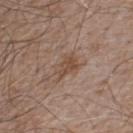Clinical impression:
This lesion was catalogued during total-body skin photography and was not selected for biopsy.
Image and clinical context:
Cropped from a total-body skin-imaging series; the visible field is about 15 mm. Captured under white-light illumination. The total-body-photography lesion software estimated a lesion area of about 6.5 mm² and an outline eccentricity of about 0.8 (0 = round, 1 = elongated). The analysis additionally found a lesion color around L≈48 a*≈17 b*≈27 in CIELAB, about 8 CIELAB-L* units darker than the surrounding skin, and a normalized border contrast of about 6.5. The software also gave a border-irregularity index near 4.5/10, a within-lesion color-variation index near 3.5/10, and peripheral color asymmetry of about 1. And it measured a nevus-likeness score of about 20/100 and a detector confidence of about 100 out of 100 that the crop contains a lesion. The patient is a male aged approximately 55. From the upper back.